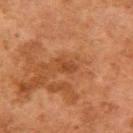workup = imaged on a skin check; not biopsied
lesion size = ≈2.5 mm
imaging modality = ~15 mm tile from a whole-body skin photo
patient = female, aged approximately 60
anatomic site = the arm
illumination = cross-polarized